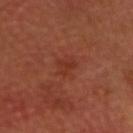{"automated_metrics": {"cielab_L": 33, "cielab_a": 28, "cielab_b": 31, "vs_skin_darker_L": 5.0, "vs_skin_contrast_norm": 5.5, "nevus_likeness_0_100": 0, "lesion_detection_confidence_0_100": 100}, "image": {"source": "total-body photography crop", "field_of_view_mm": 15}, "site": "head or neck", "lesion_size": {"long_diameter_mm_approx": 2.5}, "lighting": "cross-polarized", "patient": {"sex": "male", "age_approx": 65}}